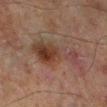Q: Was this lesion biopsied?
A: catalogued during a skin exam; not biopsied